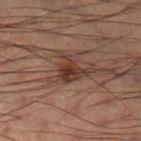Notes:
– workup: no biopsy performed (imaged during a skin exam)
– site: the left lower leg
– acquisition: 15 mm crop, total-body photography
– patient: male, approximately 50 years of age